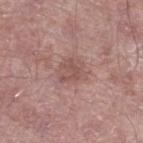Captured during whole-body skin photography for melanoma surveillance; the lesion was not biopsied. A 15 mm close-up extracted from a 3D total-body photography capture. On the left lower leg. A male patient, aged 53 to 57.